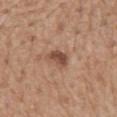Q: Is there a histopathology result?
A: total-body-photography surveillance lesion; no biopsy
Q: What are the patient's age and sex?
A: male, in their 70s
Q: What is the imaging modality?
A: ~15 mm crop, total-body skin-cancer survey
Q: Where on the body is the lesion?
A: the mid back